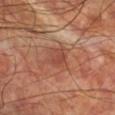{
  "biopsy_status": "not biopsied; imaged during a skin examination",
  "patient": {
    "sex": "male",
    "age_approx": 60
  },
  "image": {
    "source": "total-body photography crop",
    "field_of_view_mm": 15
  },
  "site": "right lower leg"
}